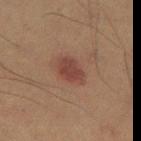Recorded during total-body skin imaging; not selected for excision or biopsy.
Longest diameter approximately 3.5 mm.
The lesion-visualizer software estimated a lesion area of about 6 mm² and an outline eccentricity of about 0.75 (0 = round, 1 = elongated). The analysis additionally found a lesion color around L≈34 a*≈19 b*≈21 in CIELAB, about 8 CIELAB-L* units darker than the surrounding skin, and a normalized border contrast of about 7.5. And it measured a border-irregularity index near 2.5/10, a color-variation rating of about 1.5/10, and peripheral color asymmetry of about 0.5.
A male subject, in their 60s.
Imaged with cross-polarized lighting.
A 15 mm close-up extracted from a 3D total-body photography capture.
The lesion is on the left thigh.